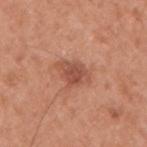Imaged during a routine full-body skin examination; the lesion was not biopsied and no histopathology is available.
Automated tile analysis of the lesion measured an outline eccentricity of about 0.6 (0 = round, 1 = elongated) and a symmetry-axis asymmetry near 0.3. It also reported a lesion color around L≈52 a*≈26 b*≈31 in CIELAB and roughly 10 lightness units darker than nearby skin. It also reported lesion-presence confidence of about 100/100.
On the right upper arm.
A close-up tile cropped from a whole-body skin photograph, about 15 mm across.
This is a white-light tile.
The subject is a male approximately 55 years of age.
The recorded lesion diameter is about 3.5 mm.